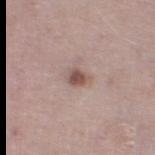The lesion was tiled from a total-body skin photograph and was not biopsied.
On the left thigh.
A male patient, aged approximately 75.
A lesion tile, about 15 mm wide, cut from a 3D total-body photograph.